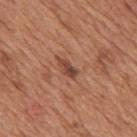tile lighting: white-light illumination | lesion diameter: about 3 mm | image source: ~15 mm crop, total-body skin-cancer survey | anatomic site: the mid back | patient: male, in their mid-60s | automated metrics: an eccentricity of roughly 0.85; a lesion color around L≈45 a*≈24 b*≈29 in CIELAB and a normalized lesion–skin contrast near 8; a border-irregularity index near 2/10 and a color-variation rating of about 4/10; a classifier nevus-likeness of about 0/100.